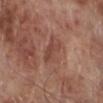Captured during whole-body skin photography for melanoma surveillance; the lesion was not biopsied. The tile uses white-light illumination. This image is a 15 mm lesion crop taken from a total-body photograph. The lesion's longest dimension is about 3 mm. Automated image analysis of the tile measured a lesion color around L≈45 a*≈23 b*≈26 in CIELAB, roughly 7 lightness units darker than nearby skin, and a normalized lesion–skin contrast near 5.5. The analysis additionally found a classifier nevus-likeness of about 0/100. The subject is a male about 70 years old. The lesion is on the right lower leg.